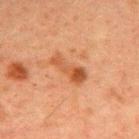Context: The patient is a male about 60 years old. The lesion's longest dimension is about 4.5 mm. The lesion is on the upper back. A 15 mm crop from a total-body photograph taken for skin-cancer surveillance.